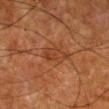Case summary:
* follow-up: imaged on a skin check; not biopsied
* image: ~15 mm crop, total-body skin-cancer survey
* anatomic site: the right lower leg
* subject: male, in their 80s
* lighting: cross-polarized